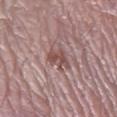Impression:
The lesion was photographed on a routine skin check and not biopsied; there is no pathology result.
Image and clinical context:
The lesion is located on the leg. A 15 mm close-up extracted from a 3D total-body photography capture. A male patient, about 75 years old. Imaged with white-light lighting. The lesion's longest dimension is about 2.5 mm.